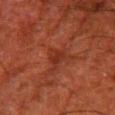Part of a total-body skin-imaging series; this lesion was reviewed on a skin check and was not flagged for biopsy.
Captured under cross-polarized illumination.
Longest diameter approximately 2.5 mm.
A male patient, aged 78 to 82.
An algorithmic analysis of the crop reported an area of roughly 4 mm² and a shape-asymmetry score of about 0.35 (0 = symmetric). It also reported a mean CIELAB color near L≈26 a*≈25 b*≈27 and about 6 CIELAB-L* units darker than the surrounding skin. It also reported a peripheral color-asymmetry measure near 1. The software also gave a nevus-likeness score of about 0/100 and lesion-presence confidence of about 85/100.
A region of skin cropped from a whole-body photographic capture, roughly 15 mm wide.
The lesion is located on the right thigh.